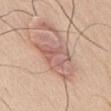Case summary:
* notes: total-body-photography surveillance lesion; no biopsy
* image: 15 mm crop, total-body photography
* tile lighting: white-light illumination
* diameter: ≈7 mm
* patient: male, roughly 55 years of age
* anatomic site: the abdomen